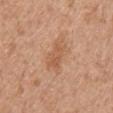follow-up: imaged on a skin check; not biopsied | subject: female, roughly 60 years of age | image source: 15 mm crop, total-body photography | diameter: ~3.5 mm (longest diameter) | anatomic site: the arm | automated lesion analysis: a lesion–skin lightness drop of about 7; border irregularity of about 3 on a 0–10 scale, a within-lesion color-variation index near 3/10, and a peripheral color-asymmetry measure near 1; an automated nevus-likeness rating near 25 out of 100 and a detector confidence of about 100 out of 100 that the crop contains a lesion.